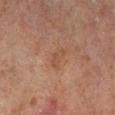workup: total-body-photography surveillance lesion; no biopsy | location: the left lower leg | lesion size: ~3 mm (longest diameter) | subject: male, aged approximately 70 | image-analysis metrics: an average lesion color of about L≈40 a*≈17 b*≈26 (CIELAB), about 5 CIELAB-L* units darker than the surrounding skin, and a lesion-to-skin contrast of about 4.5 (normalized; higher = more distinct); a border-irregularity rating of about 4/10 and radial color variation of about 0; a detector confidence of about 100 out of 100 that the crop contains a lesion | image: 15 mm crop, total-body photography | lighting: cross-polarized.